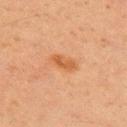The lesion was tiled from a total-body skin photograph and was not biopsied. A 15 mm crop from a total-body photograph taken for skin-cancer surveillance. The tile uses cross-polarized illumination. The patient is a male approximately 70 years of age. Approximately 3.5 mm at its widest. On the chest. Automated image analysis of the tile measured an average lesion color of about L≈49 a*≈22 b*≈36 (CIELAB) and a lesion-to-skin contrast of about 7 (normalized; higher = more distinct).